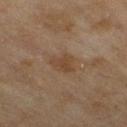- workup: catalogued during a skin exam; not biopsied
- automated lesion analysis: a footprint of about 6 mm², an outline eccentricity of about 0.75 (0 = round, 1 = elongated), and a shape-asymmetry score of about 0.3 (0 = symmetric); border irregularity of about 3 on a 0–10 scale; an automated nevus-likeness rating near 10 out of 100 and a lesion-detection confidence of about 100/100
- subject: female, in their 60s
- acquisition: ~15 mm tile from a whole-body skin photo
- location: the right thigh
- diameter: about 3.5 mm